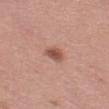This lesion was catalogued during total-body skin photography and was not selected for biopsy.
The lesion is on the left thigh.
A 15 mm close-up tile from a total-body photography series done for melanoma screening.
A female subject approximately 40 years of age.